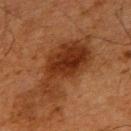Q: Was this lesion biopsied?
A: no biopsy performed (imaged during a skin exam)
Q: What lighting was used for the tile?
A: cross-polarized illumination
Q: What kind of image is this?
A: total-body-photography crop, ~15 mm field of view
Q: What is the anatomic site?
A: the back
Q: Lesion size?
A: about 8.5 mm
Q: Patient demographics?
A: male, approximately 65 years of age